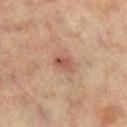<tbp_lesion>
  <biopsy_status>not biopsied; imaged during a skin examination</biopsy_status>
  <patient>
    <sex>female</sex>
    <age_approx>65</age_approx>
  </patient>
  <image>
    <source>total-body photography crop</source>
    <field_of_view_mm>15</field_of_view_mm>
  </image>
  <automated_metrics>
    <area_mm2_approx>3.5</area_mm2_approx>
    <eccentricity>0.8</eccentricity>
    <shape_asymmetry>0.2</shape_asymmetry>
    <vs_skin_darker_L>10.0</vs_skin_darker_L>
    <vs_skin_contrast_norm>7.0</vs_skin_contrast_norm>
  </automated_metrics>
  <lighting>cross-polarized</lighting>
  <site>left leg</site>
</tbp_lesion>